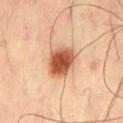acquisition = total-body-photography crop, ~15 mm field of view | lighting = cross-polarized | location = the left thigh | lesion diameter = about 4.5 mm.